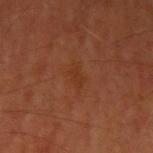| feature | finding |
|---|---|
| workup | no biopsy performed (imaged during a skin exam) |
| image source | ~15 mm tile from a whole-body skin photo |
| anatomic site | the left upper arm |
| patient | male, approximately 55 years of age |